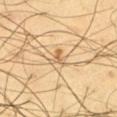biopsy_status: not biopsied; imaged during a skin examination
lighting: cross-polarized
site: left thigh
lesion_size:
  long_diameter_mm_approx: 3.0
automated_metrics:
  cielab_L: 67
  cielab_a: 16
  cielab_b: 40
  vs_skin_darker_L: 10.0
  vs_skin_contrast_norm: 6.0
patient:
  sex: male
  age_approx: 65
image:
  source: total-body photography crop
  field_of_view_mm: 15